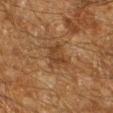Impression:
Recorded during total-body skin imaging; not selected for excision or biopsy.
Image and clinical context:
This image is a 15 mm lesion crop taken from a total-body photograph. The patient is a male about 60 years old. The lesion is located on the leg.